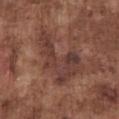Clinical impression:
Captured during whole-body skin photography for melanoma surveillance; the lesion was not biopsied.
Image and clinical context:
From the chest. Imaged with white-light lighting. A male subject approximately 75 years of age. The lesion-visualizer software estimated an outline eccentricity of about 0.8 (0 = round, 1 = elongated) and a shape-asymmetry score of about 0.65 (0 = symmetric). And it measured peripheral color asymmetry of about 1.5. It also reported an automated nevus-likeness rating near 0 out of 100. A region of skin cropped from a whole-body photographic capture, roughly 15 mm wide. Measured at roughly 7.5 mm in maximum diameter.